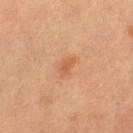Findings:
- follow-up · catalogued during a skin exam; not biopsied
- lighting · cross-polarized illumination
- patient · female, aged 43 to 47
- lesion size · ≈2.5 mm
- location · the right leg
- acquisition · 15 mm crop, total-body photography